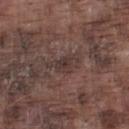| feature | finding |
|---|---|
| follow-up | catalogued during a skin exam; not biopsied |
| location | the left lower leg |
| lighting | white-light |
| acquisition | total-body-photography crop, ~15 mm field of view |
| patient | male, aged 73–77 |